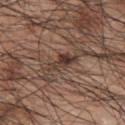The lesion was photographed on a routine skin check and not biopsied; there is no pathology result. The total-body-photography lesion software estimated a border-irregularity index near 5/10, internal color variation of about 3.5 on a 0–10 scale, and peripheral color asymmetry of about 1. The lesion is on the upper back. The patient is a male aged around 70. A lesion tile, about 15 mm wide, cut from a 3D total-body photograph.